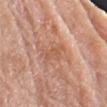A male subject aged 78–82.
An algorithmic analysis of the crop reported a footprint of about 4.5 mm², an eccentricity of roughly 0.9, and two-axis asymmetry of about 0.4. The analysis additionally found a border-irregularity rating of about 4.5/10, a within-lesion color-variation index near 1/10, and peripheral color asymmetry of about 0. It also reported a nevus-likeness score of about 0/100 and lesion-presence confidence of about 100/100.
A close-up tile cropped from a whole-body skin photograph, about 15 mm across.
The lesion is located on the arm.
The lesion's longest dimension is about 3.5 mm.
This is a white-light tile.